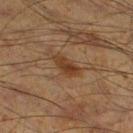Assessment: The lesion was tiled from a total-body skin photograph and was not biopsied.